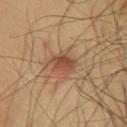{
  "biopsy_status": "not biopsied; imaged during a skin examination",
  "site": "front of the torso",
  "image": {
    "source": "total-body photography crop",
    "field_of_view_mm": 15
  },
  "patient": {
    "sex": "male",
    "age_approx": 45
  }
}